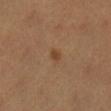workup: no biopsy performed (imaged during a skin exam); anatomic site: the right lower leg; image source: total-body-photography crop, ~15 mm field of view; patient: female, aged 38–42; lighting: cross-polarized illumination; lesion diameter: ~1.5 mm (longest diameter).